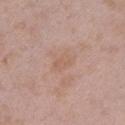Context: Imaged with white-light lighting. A male patient about 50 years old. A 15 mm crop from a total-body photograph taken for skin-cancer surveillance. The lesion's longest dimension is about 3 mm. Located on the left upper arm.